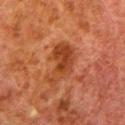biopsy status: imaged on a skin check; not biopsied
lesion size: ~5 mm (longest diameter)
patient: male, aged 78 to 82
imaging modality: total-body-photography crop, ~15 mm field of view
site: the left lower leg
TBP lesion metrics: a symmetry-axis asymmetry near 0.4; a mean CIELAB color near L≈34 a*≈24 b*≈32, roughly 8 lightness units darker than nearby skin, and a normalized border contrast of about 8; a nevus-likeness score of about 5/100
lighting: cross-polarized